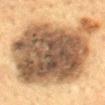Impression: The lesion was tiled from a total-body skin photograph and was not biopsied. Context: A male subject in their 60s. The recorded lesion diameter is about 13 mm. A region of skin cropped from a whole-body photographic capture, roughly 15 mm wide. Imaged with cross-polarized lighting. The lesion is located on the mid back.